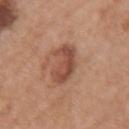Captured during whole-body skin photography for melanoma surveillance; the lesion was not biopsied. A close-up tile cropped from a whole-body skin photograph, about 15 mm across. The subject is a female in their 60s. The lesion is on the right forearm. This is a white-light tile.